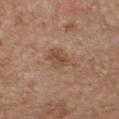{"biopsy_status": "not biopsied; imaged during a skin examination", "patient": {"sex": "female", "age_approx": 40}, "lighting": "white-light", "automated_metrics": {"color_variation_0_10": 3.0, "peripheral_color_asymmetry": 1.0, "lesion_detection_confidence_0_100": 100}, "site": "chest", "image": {"source": "total-body photography crop", "field_of_view_mm": 15}}